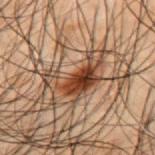Imaged during a routine full-body skin examination; the lesion was not biopsied and no histopathology is available. Automated image analysis of the tile measured a lesion area of about 14 mm², an outline eccentricity of about 0.85 (0 = round, 1 = elongated), and a shape-asymmetry score of about 0.7 (0 = symmetric). And it measured a mean CIELAB color near L≈33 a*≈17 b*≈25 and roughly 11 lightness units darker than nearby skin. And it measured a classifier nevus-likeness of about 30/100 and a lesion-detection confidence of about 100/100. Located on the mid back. A region of skin cropped from a whole-body photographic capture, roughly 15 mm wide. About 6.5 mm across. This is a cross-polarized tile. A male patient in their 50s.